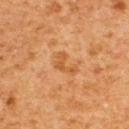biopsy status = total-body-photography surveillance lesion; no biopsy
location = the upper back
image source = ~15 mm crop, total-body skin-cancer survey
size = about 3.5 mm
patient = male, aged approximately 60